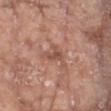workup: imaged on a skin check; not biopsied | patient: female, in their mid-70s | lesion diameter: ~2.5 mm (longest diameter) | illumination: white-light illumination | image source: ~15 mm crop, total-body skin-cancer survey | body site: the chest.